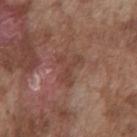Clinical impression:
Part of a total-body skin-imaging series; this lesion was reviewed on a skin check and was not flagged for biopsy.
Background:
The lesion-visualizer software estimated a footprint of about 6.5 mm², an outline eccentricity of about 0.75 (0 = round, 1 = elongated), and a shape-asymmetry score of about 0.45 (0 = symmetric). The analysis additionally found a detector confidence of about 100 out of 100 that the crop contains a lesion. Approximately 3.5 mm at its widest. A male patient roughly 75 years of age. A lesion tile, about 15 mm wide, cut from a 3D total-body photograph. The lesion is located on the chest. Captured under white-light illumination.